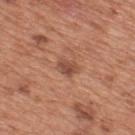The lesion was photographed on a routine skin check and not biopsied; there is no pathology result.
Located on the upper back.
Cropped from a total-body skin-imaging series; the visible field is about 15 mm.
The subject is a male in their mid-60s.
Captured under white-light illumination.